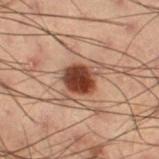  biopsy_status: not biopsied; imaged during a skin examination
  automated_metrics:
    area_mm2_approx: 8.0
    cielab_L: 32
    cielab_a: 21
    cielab_b: 25
    vs_skin_darker_L: 17.0
    vs_skin_contrast_norm: 14.0
    color_variation_0_10: 5.0
    peripheral_color_asymmetry: 1.5
    nevus_likeness_0_100: 100
  lesion_size:
    long_diameter_mm_approx: 3.5
  lighting: cross-polarized
  image:
    source: total-body photography crop
    field_of_view_mm: 15
  patient:
    sex: male
    age_approx: 55
  site: right thigh